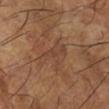notes = catalogued during a skin exam; not biopsied | imaging modality = ~15 mm tile from a whole-body skin photo | anatomic site = the left lower leg | patient = male, approximately 65 years of age.